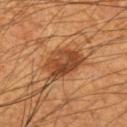{
  "biopsy_status": "not biopsied; imaged during a skin examination",
  "automated_metrics": {
    "area_mm2_approx": 12.0,
    "eccentricity": 0.7,
    "shape_asymmetry": 0.25,
    "cielab_L": 38,
    "cielab_a": 22,
    "cielab_b": 32,
    "vs_skin_darker_L": 11.0,
    "vs_skin_contrast_norm": 9.0,
    "border_irregularity_0_10": 2.5,
    "color_variation_0_10": 5.5,
    "peripheral_color_asymmetry": 2.0
  },
  "image": {
    "source": "total-body photography crop",
    "field_of_view_mm": 15
  },
  "lighting": "cross-polarized",
  "site": "right upper arm",
  "patient": {
    "sex": "male",
    "age_approx": 55
  },
  "lesion_size": {
    "long_diameter_mm_approx": 4.5
  }
}